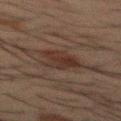{"biopsy_status": "not biopsied; imaged during a skin examination", "lesion_size": {"long_diameter_mm_approx": 4.0}, "image": {"source": "total-body photography crop", "field_of_view_mm": 15}, "lighting": "cross-polarized", "site": "mid back", "patient": {"sex": "male", "age_approx": 50}}